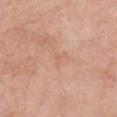Q: Is there a histopathology result?
A: no biopsy performed (imaged during a skin exam)